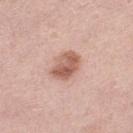follow-up: total-body-photography surveillance lesion; no biopsy | patient: female, aged 38 to 42 | site: the leg | image: 15 mm crop, total-body photography.